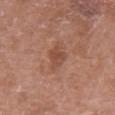Clinical impression:
Part of a total-body skin-imaging series; this lesion was reviewed on a skin check and was not flagged for biopsy.
Background:
This is a white-light tile. Approximately 3 mm at its widest. Cropped from a whole-body photographic skin survey; the tile spans about 15 mm. A female subject, aged around 70. Located on the front of the torso.